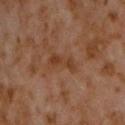Impression:
Captured during whole-body skin photography for melanoma surveillance; the lesion was not biopsied.
Acquisition and patient details:
Cropped from a total-body skin-imaging series; the visible field is about 15 mm. Captured under cross-polarized illumination. The lesion's longest dimension is about 3.5 mm. From the front of the torso. A male patient, in their 60s.